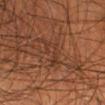Impression: Captured during whole-body skin photography for melanoma surveillance; the lesion was not biopsied. Acquisition and patient details: Captured under cross-polarized illumination. Automated tile analysis of the lesion measured a lesion area of about 4 mm². The recorded lesion diameter is about 3.5 mm. On the right lower leg. A region of skin cropped from a whole-body photographic capture, roughly 15 mm wide. A male patient roughly 55 years of age.